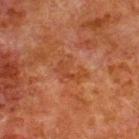No biopsy was performed on this lesion — it was imaged during a full skin examination and was not determined to be concerning.
This image is a 15 mm lesion crop taken from a total-body photograph.
The recorded lesion diameter is about 3.5 mm.
The tile uses cross-polarized illumination.
From the upper back.
A male subject, aged 78–82.